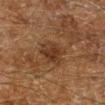No biopsy was performed on this lesion — it was imaged during a full skin examination and was not determined to be concerning. The lesion is located on the right lower leg. The patient is a male in their 60s. Imaged with cross-polarized lighting. Measured at roughly 3.5 mm in maximum diameter. A region of skin cropped from a whole-body photographic capture, roughly 15 mm wide.